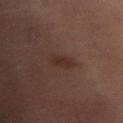biopsy status=total-body-photography surveillance lesion; no biopsy